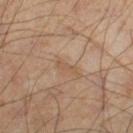follow-up: imaged on a skin check; not biopsied | tile lighting: cross-polarized illumination | acquisition: ~15 mm tile from a whole-body skin photo | diameter: ≈2.5 mm | anatomic site: the right lower leg | subject: male, in their mid- to late 40s.